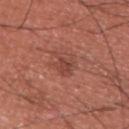notes = catalogued during a skin exam; not biopsied
anatomic site = the upper back
patient = male, in their mid- to late 30s
image = ~15 mm crop, total-body skin-cancer survey